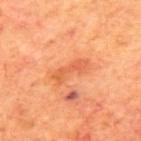workup=total-body-photography surveillance lesion; no biopsy | image=total-body-photography crop, ~15 mm field of view | site=the mid back | subject=male, about 70 years old.